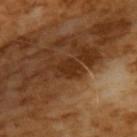Part of a total-body skin-imaging series; this lesion was reviewed on a skin check and was not flagged for biopsy. Approximately 3.5 mm at its widest. An algorithmic analysis of the crop reported a footprint of about 6 mm² and two-axis asymmetry of about 0.25. The software also gave a lesion color around L≈30 a*≈21 b*≈31 in CIELAB, roughly 8 lightness units darker than nearby skin, and a normalized lesion–skin contrast near 8. The analysis additionally found an automated nevus-likeness rating near 5 out of 100 and lesion-presence confidence of about 95/100. Captured under cross-polarized illumination. Cropped from a total-body skin-imaging series; the visible field is about 15 mm. A male subject about 65 years old.